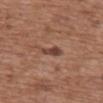The lesion was tiled from a total-body skin photograph and was not biopsied. On the upper back. Cropped from a whole-body photographic skin survey; the tile spans about 15 mm. Longest diameter approximately 3 mm. Automated tile analysis of the lesion measured a lesion color around L≈43 a*≈21 b*≈25 in CIELAB, about 11 CIELAB-L* units darker than the surrounding skin, and a normalized lesion–skin contrast near 8.5. The software also gave an automated nevus-likeness rating near 10 out of 100 and lesion-presence confidence of about 100/100. A female patient about 75 years old.